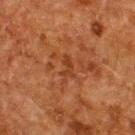Recorded during total-body skin imaging; not selected for excision or biopsy. A 15 mm close-up extracted from a 3D total-body photography capture. A male subject aged 58–62. From the upper back.